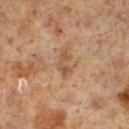Case summary:
- follow-up · catalogued during a skin exam; not biopsied
- imaging modality · ~15 mm crop, total-body skin-cancer survey
- patient · male, aged 58 to 62
- location · the left lower leg
- automated lesion analysis · a footprint of about 3.5 mm², an eccentricity of roughly 0.85, and a shape-asymmetry score of about 0.45 (0 = symmetric); a border-irregularity rating of about 6/10 and a peripheral color-asymmetry measure near 0; a nevus-likeness score of about 0/100 and a lesion-detection confidence of about 100/100
- size · ≈3 mm
- lighting · cross-polarized